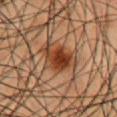anatomic site = the chest; lighting = cross-polarized illumination; acquisition = ~15 mm tile from a whole-body skin photo; subject = male, in their mid-50s; lesion diameter = ~4.5 mm (longest diameter).